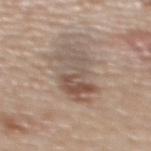Part of a total-body skin-imaging series; this lesion was reviewed on a skin check and was not flagged for biopsy.
The subject is a male approximately 70 years of age.
The recorded lesion diameter is about 6 mm.
Captured under white-light illumination.
From the upper back.
A region of skin cropped from a whole-body photographic capture, roughly 15 mm wide.